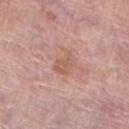| key | value |
|---|---|
| notes | total-body-photography surveillance lesion; no biopsy |
| anatomic site | the leg |
| lesion diameter | ≈2.5 mm |
| subject | female, approximately 60 years of age |
| illumination | white-light illumination |
| image | total-body-photography crop, ~15 mm field of view |